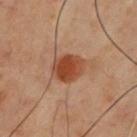notes=catalogued during a skin exam; not biopsied
site=the chest
acquisition=total-body-photography crop, ~15 mm field of view
subject=male, aged 53 to 57